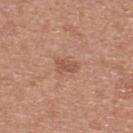{"biopsy_status": "not biopsied; imaged during a skin examination", "site": "back", "patient": {"sex": "male", "age_approx": 30}, "image": {"source": "total-body photography crop", "field_of_view_mm": 15}}